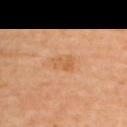workup = imaged on a skin check; not biopsied | body site = the upper back | acquisition = total-body-photography crop, ~15 mm field of view | patient = female, approximately 60 years of age.